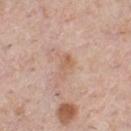Q: Is there a histopathology result?
A: imaged on a skin check; not biopsied
Q: What lighting was used for the tile?
A: white-light illumination
Q: What are the patient's age and sex?
A: male, roughly 40 years of age
Q: Lesion location?
A: the chest
Q: What is the lesion's diameter?
A: ~3 mm (longest diameter)
Q: What kind of image is this?
A: total-body-photography crop, ~15 mm field of view
Q: Automated lesion metrics?
A: an average lesion color of about L≈61 a*≈19 b*≈30 (CIELAB), roughly 7 lightness units darker than nearby skin, and a normalized lesion–skin contrast near 5.5